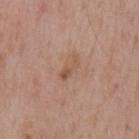Findings:
- follow-up · imaged on a skin check; not biopsied
- image · ~15 mm tile from a whole-body skin photo
- lighting · white-light illumination
- patient · male, about 55 years old
- body site · the mid back
- TBP lesion metrics · a lesion area of about 2.5 mm², an eccentricity of roughly 0.95, and two-axis asymmetry of about 0.5; a normalized lesion–skin contrast near 6; a nevus-likeness score of about 0/100